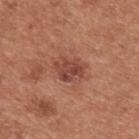Part of a total-body skin-imaging series; this lesion was reviewed on a skin check and was not flagged for biopsy. Automated image analysis of the tile measured an outline eccentricity of about 0.6 (0 = round, 1 = elongated) and two-axis asymmetry of about 0.3. It also reported an automated nevus-likeness rating near 5 out of 100 and lesion-presence confidence of about 100/100. A male patient, aged 53 to 57. Cropped from a whole-body photographic skin survey; the tile spans about 15 mm. This is a white-light tile. The lesion is on the upper back.